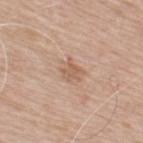notes: imaged on a skin check; not biopsied
automated lesion analysis: an area of roughly 4 mm², a shape eccentricity near 0.6, and a shape-asymmetry score of about 0.4 (0 = symmetric)
acquisition: ~15 mm crop, total-body skin-cancer survey
tile lighting: white-light
size: ~3 mm (longest diameter)
anatomic site: the upper back
patient: male, about 65 years old